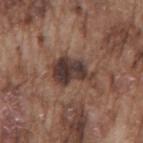The lesion was tiled from a total-body skin photograph and was not biopsied.
A male subject in their mid-70s.
Automated tile analysis of the lesion measured a footprint of about 12 mm², an eccentricity of roughly 0.8, and two-axis asymmetry of about 0.45. It also reported a mean CIELAB color near L≈37 a*≈16 b*≈20, a lesion–skin lightness drop of about 13, and a normalized border contrast of about 11.5. It also reported lesion-presence confidence of about 100/100.
Approximately 5.5 mm at its widest.
The lesion is located on the mid back.
Cropped from a total-body skin-imaging series; the visible field is about 15 mm.